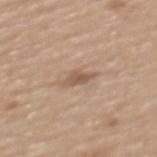follow-up: imaged on a skin check; not biopsied | anatomic site: the upper back | size: ≈3.5 mm | tile lighting: white-light | patient: female, aged around 70 | image source: ~15 mm tile from a whole-body skin photo.